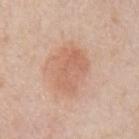Q: Was this lesion biopsied?
A: catalogued during a skin exam; not biopsied
Q: What kind of image is this?
A: total-body-photography crop, ~15 mm field of view
Q: What did automated image analysis measure?
A: a lesion area of about 16 mm², a shape eccentricity near 0.7, and a symmetry-axis asymmetry near 0.2
Q: How was the tile lit?
A: white-light illumination
Q: Patient demographics?
A: male, aged approximately 75
Q: Lesion location?
A: the left upper arm